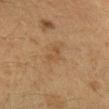{
  "biopsy_status": "not biopsied; imaged during a skin examination"
}